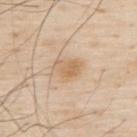{
  "biopsy_status": "not biopsied; imaged during a skin examination",
  "site": "upper back",
  "lighting": "white-light",
  "image": {
    "source": "total-body photography crop",
    "field_of_view_mm": 15
  },
  "patient": {
    "sex": "male",
    "age_approx": 80
  },
  "lesion_size": {
    "long_diameter_mm_approx": 3.5
  },
  "automated_metrics": {
    "border_irregularity_0_10": 3.0,
    "color_variation_0_10": 3.5,
    "peripheral_color_asymmetry": 1.0,
    "lesion_detection_confidence_0_100": 100
  }
}